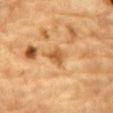{"biopsy_status": "not biopsied; imaged during a skin examination", "lesion_size": {"long_diameter_mm_approx": 2.5}, "patient": {"sex": "male", "age_approx": "85+"}, "lighting": "cross-polarized", "automated_metrics": {"area_mm2_approx": 4.5, "eccentricity": 0.6, "shape_asymmetry": 0.3, "cielab_L": 50, "cielab_a": 20, "cielab_b": 38, "vs_skin_darker_L": 9.0, "vs_skin_contrast_norm": 7.0, "nevus_likeness_0_100": 0, "lesion_detection_confidence_0_100": 100}, "image": {"source": "total-body photography crop", "field_of_view_mm": 15}, "site": "front of the torso"}